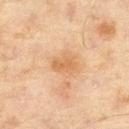  biopsy_status: not biopsied; imaged during a skin examination
  image:
    source: total-body photography crop
    field_of_view_mm: 15
  lesion_size:
    long_diameter_mm_approx: 2.5
  automated_metrics:
    area_mm2_approx: 4.5
    eccentricity: 0.75
    cielab_L: 61
    cielab_a: 21
    cielab_b: 39
    vs_skin_darker_L: 8.0
    vs_skin_contrast_norm: 6.0
  patient:
    sex: male
    age_approx: 60
  site: leg